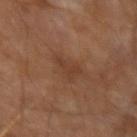Q: What are the patient's age and sex?
A: male, in their mid-60s
Q: What kind of image is this?
A: ~15 mm tile from a whole-body skin photo
Q: Where on the body is the lesion?
A: the right upper arm